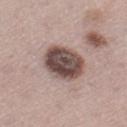Recorded during total-body skin imaging; not selected for excision or biopsy. The tile uses white-light illumination. A male subject, in their mid- to late 60s. Located on the left thigh. Measured at roughly 5 mm in maximum diameter. A roughly 15 mm field-of-view crop from a total-body skin photograph.